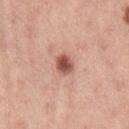biopsy status — imaged on a skin check; not biopsied
automated metrics — an area of roughly 4.5 mm², an eccentricity of roughly 0.6, and two-axis asymmetry of about 0.2; roughly 16 lightness units darker than nearby skin and a lesion-to-skin contrast of about 10.5 (normalized; higher = more distinct); a classifier nevus-likeness of about 95/100
tile lighting — white-light illumination
subject — female, in their mid-20s
lesion diameter — ~2.5 mm (longest diameter)
image — ~15 mm crop, total-body skin-cancer survey
site — the right thigh